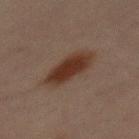Q: Was a biopsy performed?
A: total-body-photography surveillance lesion; no biopsy
Q: How was this image acquired?
A: ~15 mm tile from a whole-body skin photo
Q: What is the lesion's diameter?
A: about 5.5 mm
Q: Automated lesion metrics?
A: a lesion color around L≈28 a*≈15 b*≈21 in CIELAB and a normalized lesion–skin contrast near 9.5; an automated nevus-likeness rating near 100 out of 100 and a lesion-detection confidence of about 100/100
Q: Patient demographics?
A: male, aged around 30
Q: What is the anatomic site?
A: the mid back